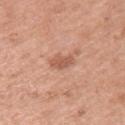<lesion>
  <biopsy_status>not biopsied; imaged during a skin examination</biopsy_status>
  <image>
    <source>total-body photography crop</source>
    <field_of_view_mm>15</field_of_view_mm>
  </image>
  <patient>
    <sex>female</sex>
    <age_approx>50</age_approx>
  </patient>
  <lighting>white-light</lighting>
  <site>arm</site>
</lesion>